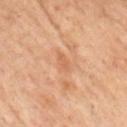Part of a total-body skin-imaging series; this lesion was reviewed on a skin check and was not flagged for biopsy. A male patient, aged 48 to 52. The tile uses cross-polarized illumination. About 2.5 mm across. Automated image analysis of the tile measured an area of roughly 3 mm², an outline eccentricity of about 0.9 (0 = round, 1 = elongated), and a shape-asymmetry score of about 0.3 (0 = symmetric). The analysis additionally found a color-variation rating of about 1/10 and radial color variation of about 0. The software also gave an automated nevus-likeness rating near 0 out of 100 and a lesion-detection confidence of about 100/100. Located on the front of the torso. A region of skin cropped from a whole-body photographic capture, roughly 15 mm wide.